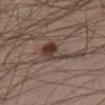No biopsy was performed on this lesion — it was imaged during a full skin examination and was not determined to be concerning. The total-body-photography lesion software estimated a footprint of about 6.5 mm² and a shape eccentricity near 0.85. A 15 mm close-up extracted from a 3D total-body photography capture. About 4.5 mm across. The lesion is located on the right lower leg. The subject is a male about 40 years old.